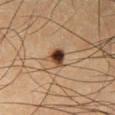automated metrics: an eccentricity of roughly 0.55 and a symmetry-axis asymmetry near 0.35; a mean CIELAB color near L≈37 a*≈17 b*≈28, about 17 CIELAB-L* units darker than the surrounding skin, and a lesion-to-skin contrast of about 13.5 (normalized; higher = more distinct) | imaging modality: total-body-photography crop, ~15 mm field of view | patient: male, aged around 35 | tile lighting: cross-polarized | size: ≈2.5 mm | body site: the left lower leg.